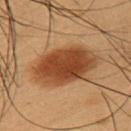This lesion was catalogued during total-body skin photography and was not selected for biopsy.
This image is a 15 mm lesion crop taken from a total-body photograph.
The subject is a male aged around 55.
Located on the right upper arm.
Imaged with cross-polarized lighting.
The lesion's longest dimension is about 7 mm.